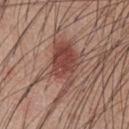This lesion was catalogued during total-body skin photography and was not selected for biopsy.
A male patient aged approximately 60.
Approximately 7.5 mm at its widest.
From the chest.
The tile uses white-light illumination.
Automated image analysis of the tile measured an area of roughly 15 mm² and a symmetry-axis asymmetry near 0.4.
A lesion tile, about 15 mm wide, cut from a 3D total-body photograph.